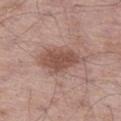Q: Was a biopsy performed?
A: catalogued during a skin exam; not biopsied
Q: What kind of image is this?
A: ~15 mm crop, total-body skin-cancer survey
Q: Lesion size?
A: about 6 mm
Q: How was the tile lit?
A: white-light illumination
Q: What are the patient's age and sex?
A: male, in their 50s
Q: Lesion location?
A: the left thigh